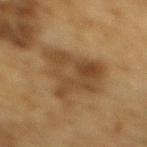No biopsy was performed on this lesion — it was imaged during a full skin examination and was not determined to be concerning.
Captured under cross-polarized illumination.
Located on the mid back.
An algorithmic analysis of the crop reported internal color variation of about 4.5 on a 0–10 scale and radial color variation of about 1.5. And it measured a classifier nevus-likeness of about 0/100.
A 15 mm crop from a total-body photograph taken for skin-cancer surveillance.
A male subject in their mid- to late 80s or older.